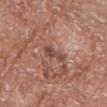Assessment:
Captured during whole-body skin photography for melanoma surveillance; the lesion was not biopsied.
Context:
Captured under white-light illumination. Approximately 3 mm at its widest. The lesion-visualizer software estimated a footprint of about 4 mm², a shape eccentricity near 0.8, and a shape-asymmetry score of about 0.25 (0 = symmetric). The software also gave a mean CIELAB color near L≈47 a*≈21 b*≈25, about 9 CIELAB-L* units darker than the surrounding skin, and a lesion-to-skin contrast of about 7 (normalized; higher = more distinct). The software also gave a color-variation rating of about 2/10 and peripheral color asymmetry of about 0.5. And it measured a lesion-detection confidence of about 100/100. A male subject, roughly 80 years of age. A roughly 15 mm field-of-view crop from a total-body skin photograph. Located on the right forearm.